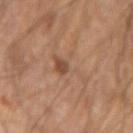Assessment: The lesion was photographed on a routine skin check and not biopsied; there is no pathology result. Context: A lesion tile, about 15 mm wide, cut from a 3D total-body photograph. Located on the arm. A male patient, roughly 65 years of age.